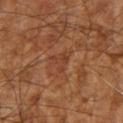Recorded during total-body skin imaging; not selected for excision or biopsy. A 15 mm crop from a total-body photograph taken for skin-cancer surveillance. The subject is aged 63–67. The lesion is located on the right upper arm.